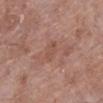Imaged during a routine full-body skin examination; the lesion was not biopsied and no histopathology is available. The patient is a female about 75 years old. Automated tile analysis of the lesion measured an eccentricity of roughly 0.85. It also reported a color-variation rating of about 1.5/10 and peripheral color asymmetry of about 0.5. The tile uses white-light illumination. About 3 mm across. A 15 mm close-up extracted from a 3D total-body photography capture. From the left lower leg.